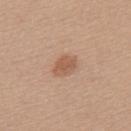Recorded during total-body skin imaging; not selected for excision or biopsy.